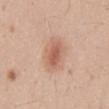Impression: No biopsy was performed on this lesion — it was imaged during a full skin examination and was not determined to be concerning. Background: A roughly 15 mm field-of-view crop from a total-body skin photograph. A male subject aged 23 to 27. On the mid back.